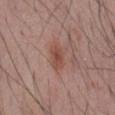| field | value |
|---|---|
| biopsy status | imaged on a skin check; not biopsied |
| patient | male, aged 53 to 57 |
| imaging modality | ~15 mm tile from a whole-body skin photo |
| anatomic site | the back |